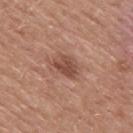Q: Was this lesion biopsied?
A: no biopsy performed (imaged during a skin exam)
Q: Lesion size?
A: ≈3.5 mm
Q: How was the tile lit?
A: white-light
Q: Lesion location?
A: the mid back
Q: What kind of image is this?
A: 15 mm crop, total-body photography
Q: Who is the patient?
A: male, approximately 50 years of age